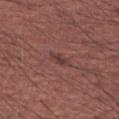Q: Was this lesion biopsied?
A: imaged on a skin check; not biopsied
Q: What lighting was used for the tile?
A: white-light illumination
Q: What is the imaging modality?
A: ~15 mm crop, total-body skin-cancer survey
Q: Where on the body is the lesion?
A: the left forearm
Q: How large is the lesion?
A: ~2.5 mm (longest diameter)
Q: What are the patient's age and sex?
A: male, aged 43–47